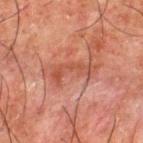Impression: Captured during whole-body skin photography for melanoma surveillance; the lesion was not biopsied. Image and clinical context: This is a cross-polarized tile. A 15 mm close-up extracted from a 3D total-body photography capture. From the upper back. An algorithmic analysis of the crop reported a footprint of about 5.5 mm², a shape eccentricity near 0.95, and two-axis asymmetry of about 0.6. The software also gave roughly 7 lightness units darker than nearby skin and a normalized lesion–skin contrast near 6. It also reported a border-irregularity index near 9/10, internal color variation of about 1 on a 0–10 scale, and radial color variation of about 0. The software also gave an automated nevus-likeness rating near 0 out of 100 and a lesion-detection confidence of about 95/100. About 5 mm across. The subject is a male approximately 50 years of age.